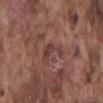Clinical impression: No biopsy was performed on this lesion — it was imaged during a full skin examination and was not determined to be concerning. Image and clinical context: From the mid back. Imaged with white-light lighting. This image is a 15 mm lesion crop taken from a total-body photograph. The lesion's longest dimension is about 3.5 mm. A male subject aged around 75.